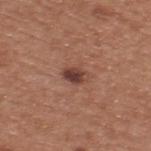Impression:
No biopsy was performed on this lesion — it was imaged during a full skin examination and was not determined to be concerning.
Context:
A lesion tile, about 15 mm wide, cut from a 3D total-body photograph. Automated tile analysis of the lesion measured a lesion-to-skin contrast of about 9.5 (normalized; higher = more distinct). The software also gave a within-lesion color-variation index near 4.5/10 and radial color variation of about 1.5. And it measured an automated nevus-likeness rating near 90 out of 100 and a detector confidence of about 100 out of 100 that the crop contains a lesion. A male subject in their mid-50s. The lesion is located on the upper back. Imaged with white-light lighting.